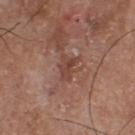workup = imaged on a skin check; not biopsied | lighting = white-light | site = the chest | image source = total-body-photography crop, ~15 mm field of view | diameter = about 3 mm | patient = male, approximately 75 years of age.